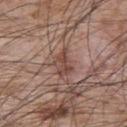| field | value |
|---|---|
| biopsy status | imaged on a skin check; not biopsied |
| image source | total-body-photography crop, ~15 mm field of view |
| patient | male, about 65 years old |
| tile lighting | white-light |
| body site | the upper back |